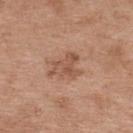Findings:
* biopsy status — no biopsy performed (imaged during a skin exam)
* site — the upper back
* lesion diameter — ≈4 mm
* image — 15 mm crop, total-body photography
* patient — female, approximately 40 years of age
* automated metrics — a footprint of about 8.5 mm², a shape eccentricity near 0.6, and a symmetry-axis asymmetry near 0.5; a lesion color around L≈53 a*≈22 b*≈30 in CIELAB, about 9 CIELAB-L* units darker than the surrounding skin, and a normalized border contrast of about 6; a border-irregularity rating of about 6/10, a color-variation rating of about 3.5/10, and radial color variation of about 1.5; a nevus-likeness score of about 5/100 and lesion-presence confidence of about 100/100